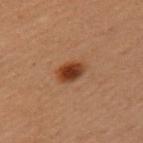Q: What is the anatomic site?
A: the left upper arm
Q: What is the imaging modality?
A: ~15 mm tile from a whole-body skin photo
Q: Lesion size?
A: ≈3 mm
Q: Who is the patient?
A: female, aged around 40
Q: How was the tile lit?
A: cross-polarized